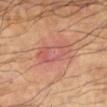| field | value |
|---|---|
| workup | total-body-photography surveillance lesion; no biopsy |
| site | the left lower leg |
| subject | male, aged around 60 |
| illumination | cross-polarized |
| acquisition | ~15 mm crop, total-body skin-cancer survey |
| automated metrics | a border-irregularity index near 3.5/10 |